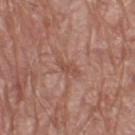Assessment:
The lesion was photographed on a routine skin check and not biopsied; there is no pathology result.
Image and clinical context:
From the left thigh. A male subject, roughly 70 years of age. Cropped from a whole-body photographic skin survey; the tile spans about 15 mm.